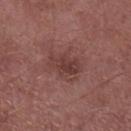Findings:
* biopsy status · total-body-photography surveillance lesion; no biopsy